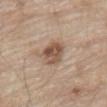The lesion was photographed on a routine skin check and not biopsied; there is no pathology result.
Cropped from a total-body skin-imaging series; the visible field is about 15 mm.
Measured at roughly 4 mm in maximum diameter.
The tile uses white-light illumination.
A male patient, aged 78 to 82.
The lesion is located on the abdomen.